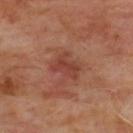Captured during whole-body skin photography for melanoma surveillance; the lesion was not biopsied. A close-up tile cropped from a whole-body skin photograph, about 15 mm across. The tile uses cross-polarized illumination. Located on the upper back. A male subject aged around 65.